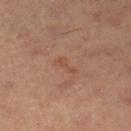Q: How was this image acquired?
A: ~15 mm crop, total-body skin-cancer survey
Q: Lesion size?
A: ≈3 mm
Q: What is the anatomic site?
A: the left lower leg
Q: Illumination type?
A: cross-polarized
Q: What are the patient's age and sex?
A: female, aged approximately 40